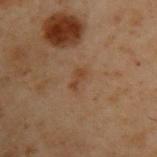workup: total-body-photography surveillance lesion; no biopsy
subject: male, approximately 55 years of age
image: total-body-photography crop, ~15 mm field of view
location: the upper back